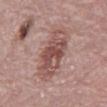* workup: catalogued during a skin exam; not biopsied
* body site: the mid back
* acquisition: total-body-photography crop, ~15 mm field of view
* patient: male, about 70 years old